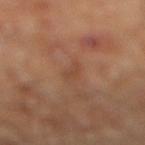Q: Is there a histopathology result?
A: total-body-photography surveillance lesion; no biopsy
Q: How was this image acquired?
A: ~15 mm tile from a whole-body skin photo
Q: How was the tile lit?
A: cross-polarized
Q: What are the patient's age and sex?
A: male, aged 68 to 72
Q: Automated lesion metrics?
A: a lesion area of about 2.5 mm² and a shape eccentricity near 0.9; a lesion color around L≈43 a*≈21 b*≈30 in CIELAB and about 6 CIELAB-L* units darker than the surrounding skin; border irregularity of about 3.5 on a 0–10 scale, internal color variation of about 0 on a 0–10 scale, and radial color variation of about 0
Q: What is the anatomic site?
A: the right lower leg
Q: How large is the lesion?
A: ~3 mm (longest diameter)